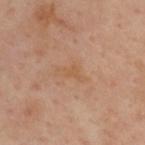{"biopsy_status": "not biopsied; imaged during a skin examination", "lesion_size": {"long_diameter_mm_approx": 3.0}, "automated_metrics": {"area_mm2_approx": 3.0, "eccentricity": 0.85, "shape_asymmetry": 0.55, "cielab_L": 56, "cielab_a": 20, "cielab_b": 34, "vs_skin_darker_L": 5.0, "vs_skin_contrast_norm": 5.0}, "lighting": "cross-polarized", "patient": {"sex": "male", "age_approx": 55}, "image": {"source": "total-body photography crop", "field_of_view_mm": 15}, "site": "upper back"}